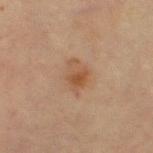No biopsy was performed on this lesion — it was imaged during a full skin examination and was not determined to be concerning. From the left thigh. The patient is a female aged 68–72. A close-up tile cropped from a whole-body skin photograph, about 15 mm across.